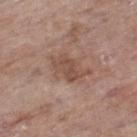Q: Was this lesion biopsied?
A: no biopsy performed (imaged during a skin exam)
Q: Lesion location?
A: the right lower leg
Q: What are the patient's age and sex?
A: female, in their mid- to late 80s or older
Q: Automated lesion metrics?
A: a lesion color around L≈49 a*≈19 b*≈26 in CIELAB, about 8 CIELAB-L* units darker than the surrounding skin, and a normalized lesion–skin contrast near 6.5; a border-irregularity index near 4/10, internal color variation of about 2.5 on a 0–10 scale, and radial color variation of about 1
Q: Illumination type?
A: white-light
Q: Lesion size?
A: ~4.5 mm (longest diameter)
Q: What is the imaging modality?
A: ~15 mm tile from a whole-body skin photo